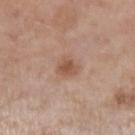Findings:
• workup · total-body-photography surveillance lesion; no biopsy
• image source · ~15 mm crop, total-body skin-cancer survey
• tile lighting · white-light illumination
• patient · male, approximately 55 years of age
• lesion size · ≈2.5 mm
• image-analysis metrics · a mean CIELAB color near L≈53 a*≈20 b*≈30 and a normalized lesion–skin contrast near 7.5; a nevus-likeness score of about 25/100
• site · the left upper arm